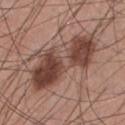A 15 mm crop from a total-body photograph taken for skin-cancer surveillance. Automated tile analysis of the lesion measured a mean CIELAB color near L≈43 a*≈20 b*≈24 and a lesion–skin lightness drop of about 14. The software also gave a border-irregularity rating of about 5.5/10. The analysis additionally found a classifier nevus-likeness of about 75/100 and a detector confidence of about 100 out of 100 that the crop contains a lesion. Located on the chest. The tile uses white-light illumination. A male subject, roughly 30 years of age. Approximately 9 mm at its widest.